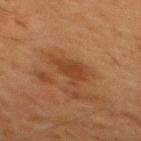| feature | finding |
|---|---|
| workup | no biopsy performed (imaged during a skin exam) |
| patient | male, aged approximately 65 |
| anatomic site | the back |
| lesion diameter | ~4 mm (longest diameter) |
| illumination | cross-polarized illumination |
| imaging modality | ~15 mm crop, total-body skin-cancer survey |